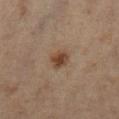Case summary:
– notes · total-body-photography surveillance lesion; no biopsy
– automated lesion analysis · a mean CIELAB color near L≈44 a*≈17 b*≈30, roughly 10 lightness units darker than nearby skin, and a lesion-to-skin contrast of about 8.5 (normalized; higher = more distinct); a border-irregularity index near 1.5/10, internal color variation of about 3.5 on a 0–10 scale, and radial color variation of about 1; lesion-presence confidence of about 100/100
– site · the left lower leg
– image · total-body-photography crop, ~15 mm field of view
– patient · female, approximately 55 years of age
– illumination · cross-polarized illumination
– diameter · ~2.5 mm (longest diameter)